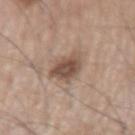The lesion is located on the left upper arm.
A lesion tile, about 15 mm wide, cut from a 3D total-body photograph.
The lesion's longest dimension is about 4.5 mm.
The tile uses white-light illumination.
The subject is a male aged around 75.